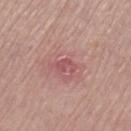{"biopsy_status": "not biopsied; imaged during a skin examination", "site": "left thigh", "image": {"source": "total-body photography crop", "field_of_view_mm": 15}, "patient": {"sex": "female", "age_approx": 70}, "lighting": "white-light", "lesion_size": {"long_diameter_mm_approx": 2.5}}